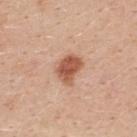Clinical impression:
Recorded during total-body skin imaging; not selected for excision or biopsy.
Acquisition and patient details:
Located on the upper back. Cropped from a total-body skin-imaging series; the visible field is about 15 mm. A female subject aged approximately 40. An algorithmic analysis of the crop reported a lesion area of about 8.5 mm², an eccentricity of roughly 0.65, and two-axis asymmetry of about 0.3. It also reported about 13 CIELAB-L* units darker than the surrounding skin and a lesion-to-skin contrast of about 9 (normalized; higher = more distinct). The analysis additionally found a border-irregularity rating of about 2.5/10, a within-lesion color-variation index near 4/10, and peripheral color asymmetry of about 1. The software also gave an automated nevus-likeness rating near 95 out of 100 and lesion-presence confidence of about 100/100. The tile uses white-light illumination.